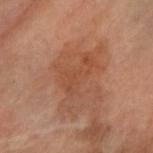Findings:
- follow-up: catalogued during a skin exam; not biopsied
- lesion size: ≈7.5 mm
- lighting: cross-polarized
- site: the arm
- imaging modality: total-body-photography crop, ~15 mm field of view
- patient: female, roughly 60 years of age